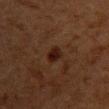Q: Was a biopsy performed?
A: total-body-photography surveillance lesion; no biopsy
Q: What is the imaging modality?
A: ~15 mm crop, total-body skin-cancer survey
Q: Where on the body is the lesion?
A: the chest
Q: Who is the patient?
A: female, approximately 50 years of age
Q: What is the lesion's diameter?
A: about 2.5 mm
Q: Automated lesion metrics?
A: an area of roughly 3.5 mm², an outline eccentricity of about 0.8 (0 = round, 1 = elongated), and two-axis asymmetry of about 0.2; a lesion color around L≈17 a*≈17 b*≈20 in CIELAB, roughly 7 lightness units darker than nearby skin, and a normalized lesion–skin contrast near 10; a within-lesion color-variation index near 3.5/10 and radial color variation of about 1; a nevus-likeness score of about 55/100 and a detector confidence of about 100 out of 100 that the crop contains a lesion
Q: How was the tile lit?
A: cross-polarized